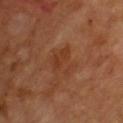- follow-up · catalogued during a skin exam; not biopsied
- lesion size · ≈3.5 mm
- body site · the front of the torso
- illumination · cross-polarized illumination
- subject · male, in their mid- to late 60s
- imaging modality · ~15 mm tile from a whole-body skin photo